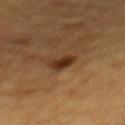notes: no biopsy performed (imaged during a skin exam) | acquisition: total-body-photography crop, ~15 mm field of view | image-analysis metrics: an area of roughly 4 mm², an eccentricity of roughly 0.75, and two-axis asymmetry of about 0.25; an average lesion color of about L≈25 a*≈17 b*≈26 (CIELAB), a lesion–skin lightness drop of about 10, and a lesion-to-skin contrast of about 11 (normalized; higher = more distinct); a classifier nevus-likeness of about 95/100 and a detector confidence of about 100 out of 100 that the crop contains a lesion | lesion diameter: ≈2.5 mm | tile lighting: cross-polarized | subject: female, approximately 55 years of age | body site: the mid back.